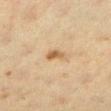Captured during whole-body skin photography for melanoma surveillance; the lesion was not biopsied. A female patient, aged around 40. From the left lower leg. Cropped from a whole-body photographic skin survey; the tile spans about 15 mm. The tile uses cross-polarized illumination. Measured at roughly 2.5 mm in maximum diameter.